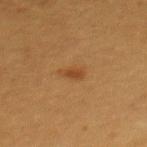Q: Was this lesion biopsied?
A: no biopsy performed (imaged during a skin exam)
Q: How was this image acquired?
A: 15 mm crop, total-body photography
Q: Lesion size?
A: ~2.5 mm (longest diameter)
Q: What are the patient's age and sex?
A: female, aged around 55
Q: Lesion location?
A: the upper back
Q: What lighting was used for the tile?
A: cross-polarized illumination